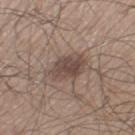No biopsy was performed on this lesion — it was imaged during a full skin examination and was not determined to be concerning. The subject is a male approximately 60 years of age. This is a white-light tile. A region of skin cropped from a whole-body photographic capture, roughly 15 mm wide. The lesion's longest dimension is about 5 mm. The lesion is on the left thigh.